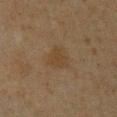The lesion was photographed on a routine skin check and not biopsied; there is no pathology result. A roughly 15 mm field-of-view crop from a total-body skin photograph. An algorithmic analysis of the crop reported a footprint of about 6 mm², an eccentricity of roughly 0.55, and a symmetry-axis asymmetry near 0.3. The software also gave roughly 4 lightness units darker than nearby skin. The analysis additionally found a classifier nevus-likeness of about 0/100. Located on the chest. A male patient, aged around 60.